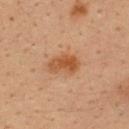Clinical impression: Captured during whole-body skin photography for melanoma surveillance; the lesion was not biopsied. Background: A male patient, aged approximately 35. The tile uses cross-polarized illumination. An algorithmic analysis of the crop reported a shape eccentricity near 0.85 and a symmetry-axis asymmetry near 0.25. It also reported an average lesion color of about L≈48 a*≈22 b*≈35 (CIELAB), about 10 CIELAB-L* units darker than the surrounding skin, and a normalized border contrast of about 8. The software also gave internal color variation of about 3.5 on a 0–10 scale. The analysis additionally found a classifier nevus-likeness of about 85/100 and a detector confidence of about 100 out of 100 that the crop contains a lesion. From the upper back. Longest diameter approximately 4 mm. A close-up tile cropped from a whole-body skin photograph, about 15 mm across.